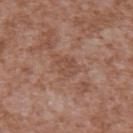notes=catalogued during a skin exam; not biopsied
subject=male, roughly 45 years of age
site=the upper back
imaging modality=~15 mm crop, total-body skin-cancer survey
diameter=~3 mm (longest diameter)
lighting=white-light illumination
automated metrics=a footprint of about 6 mm², an eccentricity of roughly 0.65, and two-axis asymmetry of about 0.35; a lesion–skin lightness drop of about 6; an automated nevus-likeness rating near 0 out of 100